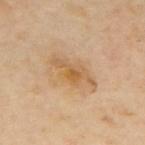Clinical impression:
The lesion was tiled from a total-body skin photograph and was not biopsied.
Acquisition and patient details:
The lesion is on the upper back. Automated tile analysis of the lesion measured an outline eccentricity of about 0.9 (0 = round, 1 = elongated) and a shape-asymmetry score of about 0.35 (0 = symmetric). The recorded lesion diameter is about 5.5 mm. A close-up tile cropped from a whole-body skin photograph, about 15 mm across. Captured under cross-polarized illumination. A male patient aged around 65.